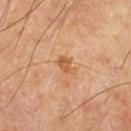Impression: No biopsy was performed on this lesion — it was imaged during a full skin examination and was not determined to be concerning. Context: The recorded lesion diameter is about 2.5 mm. On the right lower leg. A male patient, aged 63–67. A close-up tile cropped from a whole-body skin photograph, about 15 mm across.